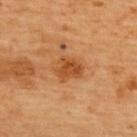Assessment: The lesion was photographed on a routine skin check and not biopsied; there is no pathology result. Image and clinical context: Automated image analysis of the tile measured a border-irregularity index near 3/10 and a within-lesion color-variation index near 3.5/10. A subject aged approximately 70. Longest diameter approximately 3.5 mm. The lesion is on the back. Cropped from a total-body skin-imaging series; the visible field is about 15 mm. Imaged with cross-polarized lighting.